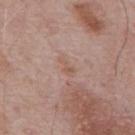Clinical impression:
No biopsy was performed on this lesion — it was imaged during a full skin examination and was not determined to be concerning.
Context:
A 15 mm crop from a total-body photograph taken for skin-cancer surveillance. The lesion is on the mid back. A male subject in their mid- to late 60s.